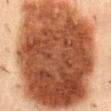Q: Is there a histopathology result?
A: catalogued during a skin exam; not biopsied
Q: Lesion location?
A: the back
Q: What kind of image is this?
A: ~15 mm crop, total-body skin-cancer survey
Q: Patient demographics?
A: male, in their mid-50s